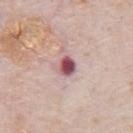Q: Lesion location?
A: the chest
Q: Illumination type?
A: white-light illumination
Q: What are the patient's age and sex?
A: male, aged 78–82
Q: What is the imaging modality?
A: 15 mm crop, total-body photography